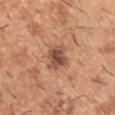The lesion was photographed on a routine skin check and not biopsied; there is no pathology result.
A male subject in their 40s.
The lesion is on the arm.
The lesion-visualizer software estimated an outline eccentricity of about 0.6 (0 = round, 1 = elongated) and two-axis asymmetry of about 0.25.
A region of skin cropped from a whole-body photographic capture, roughly 15 mm wide.
Imaged with white-light lighting.